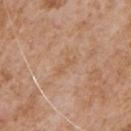This lesion was catalogued during total-body skin photography and was not selected for biopsy. About 2.5 mm across. A roughly 15 mm field-of-view crop from a total-body skin photograph. A male subject, aged 63–67. The tile uses white-light illumination. The lesion is on the chest.